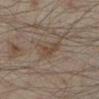| feature | finding |
|---|---|
| notes | total-body-photography surveillance lesion; no biopsy |
| imaging modality | total-body-photography crop, ~15 mm field of view |
| patient | male, aged around 45 |
| anatomic site | the left lower leg |